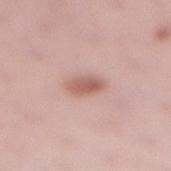The lesion is located on the right lower leg.
Cropped from a total-body skin-imaging series; the visible field is about 15 mm.
A female subject approximately 35 years of age.